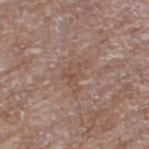Imaged during a routine full-body skin examination; the lesion was not biopsied and no histopathology is available. On the left thigh. This image is a 15 mm lesion crop taken from a total-body photograph. A female patient in their mid-70s. Captured under white-light illumination.